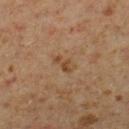Q: Was a biopsy performed?
A: no biopsy performed (imaged during a skin exam)
Q: What is the anatomic site?
A: the left lower leg
Q: Patient demographics?
A: male, approximately 60 years of age
Q: What is the lesion's diameter?
A: ~3 mm (longest diameter)
Q: What kind of image is this?
A: ~15 mm crop, total-body skin-cancer survey